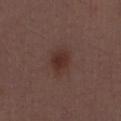<lesion>
<biopsy_status>not biopsied; imaged during a skin examination</biopsy_status>
<patient>
  <sex>male</sex>
  <age_approx>30</age_approx>
</patient>
<lighting>white-light</lighting>
<lesion_size>
  <long_diameter_mm_approx>3.0</long_diameter_mm_approx>
</lesion_size>
<site>abdomen</site>
<image>
  <source>total-body photography crop</source>
  <field_of_view_mm>15</field_of_view_mm>
</image>
</lesion>